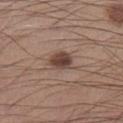Background:
The subject is a male roughly 30 years of age. This is a white-light tile. A lesion tile, about 15 mm wide, cut from a 3D total-body photograph. The lesion is on the leg. Approximately 3.5 mm at its widest.